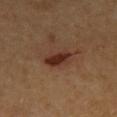Recorded during total-body skin imaging; not selected for excision or biopsy. About 4 mm across. The lesion is located on the right forearm. A lesion tile, about 15 mm wide, cut from a 3D total-body photograph. An algorithmic analysis of the crop reported a lesion area of about 7 mm² and an eccentricity of roughly 0.85. It also reported a border-irregularity rating of about 2/10, internal color variation of about 3.5 on a 0–10 scale, and a peripheral color-asymmetry measure near 1. The software also gave a nevus-likeness score of about 90/100 and lesion-presence confidence of about 100/100. A female patient roughly 55 years of age. Captured under cross-polarized illumination.